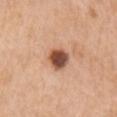Clinical impression:
The lesion was photographed on a routine skin check and not biopsied; there is no pathology result.
Background:
The total-body-photography lesion software estimated an average lesion color of about L≈50 a*≈24 b*≈31 (CIELAB), a lesion–skin lightness drop of about 19, and a lesion-to-skin contrast of about 12.5 (normalized; higher = more distinct). And it measured a border-irregularity index near 1.5/10. Located on the arm. Cropped from a total-body skin-imaging series; the visible field is about 15 mm. The subject is a female aged 53–57. The recorded lesion diameter is about 3 mm. The tile uses white-light illumination.